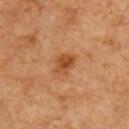A male patient about 75 years old. Located on the front of the torso. A close-up tile cropped from a whole-body skin photograph, about 15 mm across.